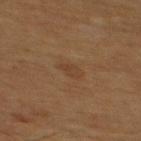The lesion's longest dimension is about 2.5 mm. Automated image analysis of the tile measured a footprint of about 3 mm², an eccentricity of roughly 0.75, and two-axis asymmetry of about 0.4. And it measured an average lesion color of about L≈36 a*≈17 b*≈29 (CIELAB), a lesion–skin lightness drop of about 5, and a normalized border contrast of about 5. Located on the mid back. The patient is a male approximately 60 years of age. A region of skin cropped from a whole-body photographic capture, roughly 15 mm wide. This is a cross-polarized tile.